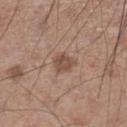Clinical summary: Cropped from a total-body skin-imaging series; the visible field is about 15 mm. From the leg. Automated image analysis of the tile measured a lesion color around L≈49 a*≈19 b*≈26 in CIELAB. The analysis additionally found a border-irregularity rating of about 2.5/10, internal color variation of about 2.5 on a 0–10 scale, and peripheral color asymmetry of about 1. The analysis additionally found an automated nevus-likeness rating near 45 out of 100. The recorded lesion diameter is about 3 mm. A male patient about 60 years old.